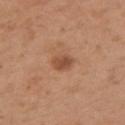| key | value |
|---|---|
| biopsy status | catalogued during a skin exam; not biopsied |
| image source | 15 mm crop, total-body photography |
| lesion diameter | about 2.5 mm |
| location | the arm |
| tile lighting | white-light illumination |
| patient | female, about 30 years old |